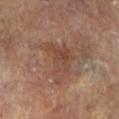The lesion was photographed on a routine skin check and not biopsied; there is no pathology result.
The subject is a female aged approximately 75.
An algorithmic analysis of the crop reported a footprint of about 13 mm² and a shape eccentricity near 0.75.
Captured under cross-polarized illumination.
A 15 mm close-up extracted from a 3D total-body photography capture.
The lesion is on the right lower leg.